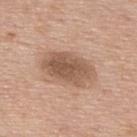<case>
<biopsy_status>not biopsied; imaged during a skin examination</biopsy_status>
<lighting>white-light</lighting>
<site>upper back</site>
<image>
  <source>total-body photography crop</source>
  <field_of_view_mm>15</field_of_view_mm>
</image>
<patient>
  <sex>male</sex>
  <age_approx>75</age_approx>
</patient>
<lesion_size>
  <long_diameter_mm_approx>6.5</long_diameter_mm_approx>
</lesion_size>
</case>